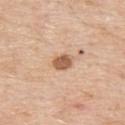Longest diameter approximately 2.5 mm. The lesion is located on the upper back. This image is a 15 mm lesion crop taken from a total-body photograph. A male subject, aged 58–62. Imaged with white-light lighting.